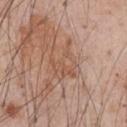– notes · catalogued during a skin exam; not biopsied
– subject · male, aged around 55
– location · the front of the torso
– image source · ~15 mm crop, total-body skin-cancer survey
– automated metrics · an area of roughly 9.5 mm², an eccentricity of roughly 0.6, and a symmetry-axis asymmetry near 0.45; about 7 CIELAB-L* units darker than the surrounding skin and a normalized border contrast of about 5.5; a classifier nevus-likeness of about 0/100
– lighting · white-light illumination